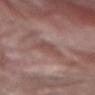body site: the right forearm
subject: female, aged 68 to 72
TBP lesion metrics: a shape eccentricity near 0.55 and a shape-asymmetry score of about 0.45 (0 = symmetric); a mean CIELAB color near L≈48 a*≈20 b*≈22, about 6 CIELAB-L* units darker than the surrounding skin, and a lesion-to-skin contrast of about 4.5 (normalized; higher = more distinct); lesion-presence confidence of about 85/100
imaging modality: total-body-photography crop, ~15 mm field of view
illumination: white-light illumination
diameter: ≈2.5 mm